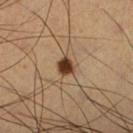Q: Was a biopsy performed?
A: catalogued during a skin exam; not biopsied
Q: How was this image acquired?
A: ~15 mm tile from a whole-body skin photo
Q: Illumination type?
A: cross-polarized illumination
Q: What are the patient's age and sex?
A: male, approximately 65 years of age
Q: How large is the lesion?
A: about 2.5 mm
Q: What is the anatomic site?
A: the right lower leg
Q: What did automated image analysis measure?
A: a lesion area of about 4 mm², an outline eccentricity of about 0.45 (0 = round, 1 = elongated), and a symmetry-axis asymmetry near 0.25; a nevus-likeness score of about 100/100 and a detector confidence of about 100 out of 100 that the crop contains a lesion